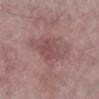Recorded during total-body skin imaging; not selected for excision or biopsy.
The tile uses white-light illumination.
Automated image analysis of the tile measured an average lesion color of about L≈48 a*≈21 b*≈17 (CIELAB) and a lesion-to-skin contrast of about 5.5 (normalized; higher = more distinct). It also reported border irregularity of about 2.5 on a 0–10 scale.
The lesion's longest dimension is about 4 mm.
This image is a 15 mm lesion crop taken from a total-body photograph.
The subject is a female aged 58 to 62.
On the left lower leg.